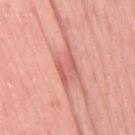workup: no biopsy performed (imaged during a skin exam)
site: the right thigh
automated lesion analysis: a footprint of about 8.5 mm², an outline eccentricity of about 0.8 (0 = round, 1 = elongated), and a symmetry-axis asymmetry near 0.3; an average lesion color of about L≈62 a*≈30 b*≈29 (CIELAB); border irregularity of about 4.5 on a 0–10 scale and internal color variation of about 4 on a 0–10 scale
lighting: white-light illumination
imaging modality: ~15 mm tile from a whole-body skin photo
size: ~5 mm (longest diameter)
patient: female, aged around 40